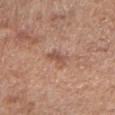Clinical impression: Imaged during a routine full-body skin examination; the lesion was not biopsied and no histopathology is available. Image and clinical context: This image is a 15 mm lesion crop taken from a total-body photograph. An algorithmic analysis of the crop reported a border-irregularity index near 4/10, internal color variation of about 0.5 on a 0–10 scale, and peripheral color asymmetry of about 0. The software also gave a nevus-likeness score of about 0/100. A female subject roughly 75 years of age. From the right forearm.